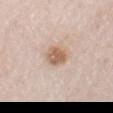The lesion was tiled from a total-body skin photograph and was not biopsied.
Located on the right thigh.
Cropped from a total-body skin-imaging series; the visible field is about 15 mm.
A male subject aged 78–82.
The recorded lesion diameter is about 3 mm.
Captured under white-light illumination.